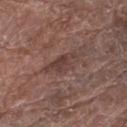notes: catalogued during a skin exam; not biopsied | location: the right lower leg | image: ~15 mm crop, total-body skin-cancer survey | patient: female, aged 78–82 | tile lighting: white-light.